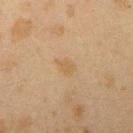This lesion was catalogued during total-body skin photography and was not selected for biopsy.